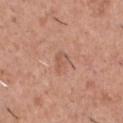No biopsy was performed on this lesion — it was imaged during a full skin examination and was not determined to be concerning.
The lesion is located on the chest.
A male patient, aged 43 to 47.
Longest diameter approximately 2.5 mm.
The total-body-photography lesion software estimated a lesion area of about 3.5 mm², an eccentricity of roughly 0.75, and a shape-asymmetry score of about 0.3 (0 = symmetric). The software also gave a lesion color around L≈56 a*≈22 b*≈30 in CIELAB.
A 15 mm crop from a total-body photograph taken for skin-cancer surveillance.
Imaged with white-light lighting.